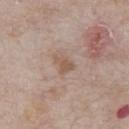workup: total-body-photography surveillance lesion; no biopsy
lighting: white-light
site: the chest
acquisition: ~15 mm crop, total-body skin-cancer survey
subject: male, approximately 65 years of age
lesion size: ≈2.5 mm
image-analysis metrics: an eccentricity of roughly 0.75 and two-axis asymmetry of about 0.4; about 8 CIELAB-L* units darker than the surrounding skin and a lesion-to-skin contrast of about 6.5 (normalized; higher = more distinct); an automated nevus-likeness rating near 5 out of 100 and a lesion-detection confidence of about 100/100